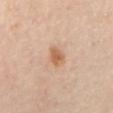The lesion was photographed on a routine skin check and not biopsied; there is no pathology result. Cropped from a whole-body photographic skin survey; the tile spans about 15 mm. Captured under cross-polarized illumination. On the mid back. A male subject aged 63–67.